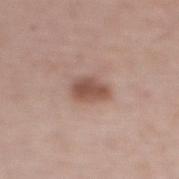The lesion was photographed on a routine skin check and not biopsied; there is no pathology result.
A lesion tile, about 15 mm wide, cut from a 3D total-body photograph.
From the upper back.
The recorded lesion diameter is about 3 mm.
Captured under white-light illumination.
A female patient, aged approximately 65.